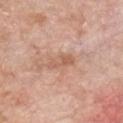From the chest. Longest diameter approximately 3.5 mm. A 15 mm close-up tile from a total-body photography series done for melanoma screening. A male patient in their 60s.